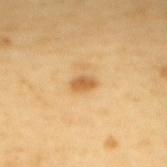workup=no biopsy performed (imaged during a skin exam); subject=female, aged 53 to 57; anatomic site=the mid back; imaging modality=total-body-photography crop, ~15 mm field of view.